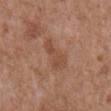notes — imaged on a skin check; not biopsied | patient — male, aged approximately 75 | acquisition — total-body-photography crop, ~15 mm field of view | location — the abdomen | illumination — white-light illumination | lesion size — ~4 mm (longest diameter).